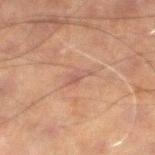The lesion was tiled from a total-body skin photograph and was not biopsied. The total-body-photography lesion software estimated a lesion area of about 2.5 mm², an eccentricity of roughly 0.9, and two-axis asymmetry of about 0.4. And it measured a normalized border contrast of about 5. The software also gave lesion-presence confidence of about 85/100. The lesion is on the left lower leg. A roughly 15 mm field-of-view crop from a total-body skin photograph. A male subject approximately 60 years of age. Measured at roughly 3 mm in maximum diameter. The tile uses cross-polarized illumination.